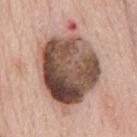{
  "biopsy_status": "not biopsied; imaged during a skin examination",
  "site": "chest",
  "image": {
    "source": "total-body photography crop",
    "field_of_view_mm": 15
  },
  "patient": {
    "sex": "male",
    "age_approx": 60
  },
  "automated_metrics": {
    "border_irregularity_0_10": 2.5,
    "color_variation_0_10": 10.0,
    "peripheral_color_asymmetry": 4.0
  },
  "lesion_size": {
    "long_diameter_mm_approx": 10.0
  },
  "lighting": "white-light"
}